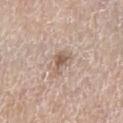Case summary:
* biopsy status · imaged on a skin check; not biopsied
* lighting · white-light illumination
* diameter · ~3 mm (longest diameter)
* image source · ~15 mm crop, total-body skin-cancer survey
* TBP lesion metrics · an average lesion color of about L≈58 a*≈16 b*≈26 (CIELAB), about 10 CIELAB-L* units darker than the surrounding skin, and a normalized lesion–skin contrast near 7; border irregularity of about 4 on a 0–10 scale and peripheral color asymmetry of about 1; a nevus-likeness score of about 55/100
* body site · the left thigh
* subject · male, approximately 80 years of age